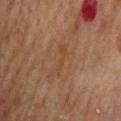| field | value |
|---|---|
| notes | imaged on a skin check; not biopsied |
| subject | male, aged 68–72 |
| body site | the mid back |
| lighting | cross-polarized illumination |
| acquisition | 15 mm crop, total-body photography |